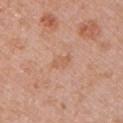No biopsy was performed on this lesion — it was imaged during a full skin examination and was not determined to be concerning. A lesion tile, about 15 mm wide, cut from a 3D total-body photograph. A female patient, roughly 50 years of age. From the left upper arm. Approximately 2.5 mm at its widest.